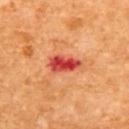workup = imaged on a skin check; not biopsied
anatomic site = the upper back
imaging modality = 15 mm crop, total-body photography
patient = female, aged around 65